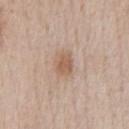Assessment: The lesion was photographed on a routine skin check and not biopsied; there is no pathology result. Clinical summary: The patient is a male about 60 years old. A lesion tile, about 15 mm wide, cut from a 3D total-body photograph. On the chest. The lesion-visualizer software estimated a footprint of about 5.5 mm², an eccentricity of roughly 0.7, and two-axis asymmetry of about 0.15. It also reported a border-irregularity index near 1.5/10, a color-variation rating of about 2/10, and peripheral color asymmetry of about 0.5. And it measured a nevus-likeness score of about 75/100 and a lesion-detection confidence of about 100/100.